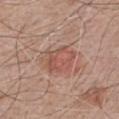{"biopsy_status": "not biopsied; imaged during a skin examination", "lesion_size": {"long_diameter_mm_approx": 4.0}, "lighting": "white-light", "image": {"source": "total-body photography crop", "field_of_view_mm": 15}, "patient": {"sex": "male", "age_approx": 65}, "site": "chest"}